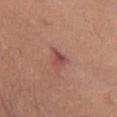Assessment:
Part of a total-body skin-imaging series; this lesion was reviewed on a skin check and was not flagged for biopsy.
Clinical summary:
The lesion is located on the chest. A 15 mm crop from a total-body photograph taken for skin-cancer surveillance. The patient is a female about 60 years old.